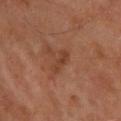This lesion was catalogued during total-body skin photography and was not selected for biopsy. A male subject, about 65 years old. A close-up tile cropped from a whole-body skin photograph, about 15 mm across. The lesion is on the upper back.